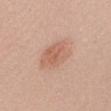Case summary:
• follow-up: total-body-photography surveillance lesion; no biopsy
• tile lighting: white-light
• automated lesion analysis: a footprint of about 12 mm² and a shape-asymmetry score of about 0.15 (0 = symmetric); a lesion color around L≈61 a*≈22 b*≈30 in CIELAB, about 8 CIELAB-L* units darker than the surrounding skin, and a normalized lesion–skin contrast near 5.5; a nevus-likeness score of about 80/100 and a detector confidence of about 100 out of 100 that the crop contains a lesion
• image: 15 mm crop, total-body photography
• lesion size: ~5 mm (longest diameter)
• subject: female, roughly 25 years of age
• location: the head or neck